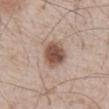  biopsy_status: not biopsied; imaged during a skin examination
  lesion_size:
    long_diameter_mm_approx: 4.5
  site: chest
  patient:
    sex: male
    age_approx: 65
  automated_metrics:
    eccentricity: 0.75
    shape_asymmetry: 0.2
    color_variation_0_10: 4.5
    peripheral_color_asymmetry: 1.5
    nevus_likeness_0_100: 100
  image:
    source: total-body photography crop
    field_of_view_mm: 15
  lighting: white-light